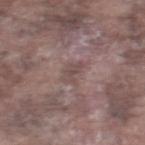Q: Was a biopsy performed?
A: catalogued during a skin exam; not biopsied
Q: How was this image acquired?
A: ~15 mm tile from a whole-body skin photo
Q: Lesion location?
A: the right thigh
Q: What are the patient's age and sex?
A: male, about 75 years old
Q: Illumination type?
A: white-light illumination
Q: How large is the lesion?
A: ≈3 mm